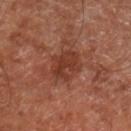biopsy_status: not biopsied; imaged during a skin examination
patient:
  age_approx: 65
image:
  source: total-body photography crop
  field_of_view_mm: 15
lighting: cross-polarized
site: right lower leg
automated_metrics:
  cielab_L: 37
  cielab_a: 25
  cielab_b: 29
  vs_skin_darker_L: 8.0
  nevus_likeness_0_100: 10
lesion_size:
  long_diameter_mm_approx: 4.0